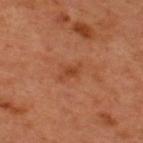This lesion was catalogued during total-body skin photography and was not selected for biopsy.